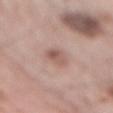Impression: Imaged during a routine full-body skin examination; the lesion was not biopsied and no histopathology is available. Image and clinical context: The lesion is located on the mid back. A male patient in their mid- to late 60s. A roughly 15 mm field-of-view crop from a total-body skin photograph. The total-body-photography lesion software estimated an average lesion color of about L≈56 a*≈20 b*≈24 (CIELAB), roughly 10 lightness units darker than nearby skin, and a normalized border contrast of about 7. The analysis additionally found a peripheral color-asymmetry measure near 2. It also reported a lesion-detection confidence of about 100/100. Measured at roughly 3.5 mm in maximum diameter. Captured under white-light illumination.